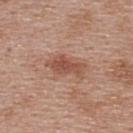Captured during whole-body skin photography for melanoma surveillance; the lesion was not biopsied.
An algorithmic analysis of the crop reported a footprint of about 8.5 mm², an eccentricity of roughly 0.85, and a symmetry-axis asymmetry near 0.35. The software also gave a nevus-likeness score of about 75/100 and a detector confidence of about 100 out of 100 that the crop contains a lesion.
On the upper back.
Imaged with white-light lighting.
A female subject, about 40 years old.
Measured at roughly 4.5 mm in maximum diameter.
A 15 mm close-up tile from a total-body photography series done for melanoma screening.